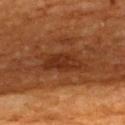  biopsy_status: not biopsied; imaged during a skin examination
  patient:
    sex: male
    age_approx: 60
  image:
    source: total-body photography crop
    field_of_view_mm: 15
  site: upper back